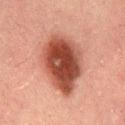Q: Is there a histopathology result?
A: imaged on a skin check; not biopsied
Q: How was the tile lit?
A: cross-polarized illumination
Q: Where on the body is the lesion?
A: the lower back
Q: Automated lesion metrics?
A: a lesion area of about 30 mm² and an eccentricity of roughly 0.65; an average lesion color of about L≈38 a*≈23 b*≈25 (CIELAB), about 15 CIELAB-L* units darker than the surrounding skin, and a lesion-to-skin contrast of about 12 (normalized; higher = more distinct); a classifier nevus-likeness of about 100/100
Q: How large is the lesion?
A: about 7.5 mm
Q: What kind of image is this?
A: total-body-photography crop, ~15 mm field of view
Q: What are the patient's age and sex?
A: male, aged around 50